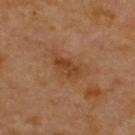Notes:
- follow-up — no biopsy performed (imaged during a skin exam)
- patient — male, roughly 70 years of age
- TBP lesion metrics — an average lesion color of about L≈40 a*≈22 b*≈34 (CIELAB), roughly 8 lightness units darker than nearby skin, and a normalized border contrast of about 7; a border-irregularity index near 3/10, a color-variation rating of about 3/10, and a peripheral color-asymmetry measure near 1
- imaging modality — 15 mm crop, total-body photography
- tile lighting — cross-polarized
- site — the upper back
- diameter — about 3.5 mm